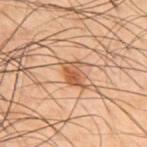biopsy_status: not biopsied; imaged during a skin examination
image:
  source: total-body photography crop
  field_of_view_mm: 15
patient:
  sex: male
  age_approx: 50
site: upper back